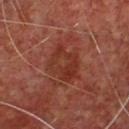Assessment: Part of a total-body skin-imaging series; this lesion was reviewed on a skin check and was not flagged for biopsy. Context: The patient is a male about 65 years old. The tile uses cross-polarized illumination. Automated image analysis of the tile measured a footprint of about 13 mm², an eccentricity of roughly 0.6, and a shape-asymmetry score of about 0.25 (0 = symmetric). And it measured a border-irregularity rating of about 3/10, internal color variation of about 4.5 on a 0–10 scale, and radial color variation of about 1.5. A 15 mm close-up tile from a total-body photography series done for melanoma screening. The recorded lesion diameter is about 5 mm. The lesion is located on the chest.